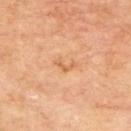biopsy_status: not biopsied; imaged during a skin examination
lighting: cross-polarized
patient:
  sex: male
  age_approx: 70
lesion_size:
  long_diameter_mm_approx: 2.5
image:
  source: total-body photography crop
  field_of_view_mm: 15
site: back